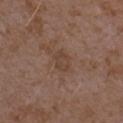Impression: No biopsy was performed on this lesion — it was imaged during a full skin examination and was not determined to be concerning. Image and clinical context: Cropped from a whole-body photographic skin survey; the tile spans about 15 mm. A female subject in their mid- to late 30s. Imaged with white-light lighting. The lesion is on the left forearm. The lesion's longest dimension is about 2.5 mm.